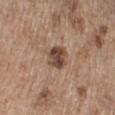Captured during whole-body skin photography for melanoma surveillance; the lesion was not biopsied. The subject is a male aged 68 to 72. Located on the right lower leg. About 2.5 mm across. A close-up tile cropped from a whole-body skin photograph, about 15 mm across. Imaged with white-light lighting.